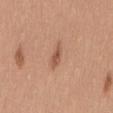workup: no biopsy performed (imaged during a skin exam) | patient: female, approximately 35 years of age | body site: the mid back | acquisition: ~15 mm crop, total-body skin-cancer survey | image-analysis metrics: border irregularity of about 3 on a 0–10 scale and a within-lesion color-variation index near 1.5/10; a nevus-likeness score of about 50/100 and a detector confidence of about 100 out of 100 that the crop contains a lesion | lighting: white-light | size: about 3 mm.